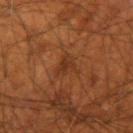The lesion was photographed on a routine skin check and not biopsied; there is no pathology result.
The lesion-visualizer software estimated an area of roughly 4.5 mm², an outline eccentricity of about 0.65 (0 = round, 1 = elongated), and a shape-asymmetry score of about 0.55 (0 = symmetric). The software also gave a border-irregularity index near 6/10, a within-lesion color-variation index near 2/10, and radial color variation of about 0.5. And it measured a detector confidence of about 100 out of 100 that the crop contains a lesion.
From the right forearm.
A region of skin cropped from a whole-body photographic capture, roughly 15 mm wide.
Longest diameter approximately 3 mm.
Captured under cross-polarized illumination.
A male patient, in their mid- to late 50s.